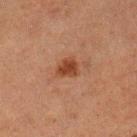workup = total-body-photography surveillance lesion; no biopsy | image source = ~15 mm crop, total-body skin-cancer survey | body site = the left lower leg | patient = female, aged 48–52 | lighting = cross-polarized illumination.